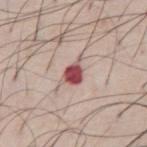Case summary:
- workup — catalogued during a skin exam; not biopsied
- patient — male, aged around 70
- diameter — ~3 mm (longest diameter)
- location — the abdomen
- acquisition — ~15 mm tile from a whole-body skin photo